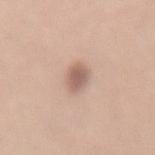Q: Automated lesion metrics?
A: a lesion–skin lightness drop of about 12 and a normalized lesion–skin contrast near 7.5
Q: What is the anatomic site?
A: the mid back
Q: Illumination type?
A: white-light
Q: What are the patient's age and sex?
A: female, aged around 35
Q: What is the imaging modality?
A: ~15 mm crop, total-body skin-cancer survey